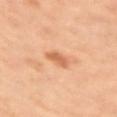Impression:
Imaged during a routine full-body skin examination; the lesion was not biopsied and no histopathology is available.
Acquisition and patient details:
A female patient aged around 35. A lesion tile, about 15 mm wide, cut from a 3D total-body photograph. Captured under cross-polarized illumination. On the right upper arm.